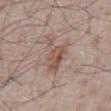image: ~15 mm crop, total-body skin-cancer survey; subject: male, aged around 65; location: the front of the torso; illumination: white-light illumination.